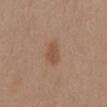notes = no biopsy performed (imaged during a skin exam); lighting = white-light illumination; acquisition = ~15 mm crop, total-body skin-cancer survey; automated lesion analysis = a mean CIELAB color near L≈51 a*≈19 b*≈30, a lesion–skin lightness drop of about 8, and a normalized border contrast of about 6.5; subject = female, approximately 55 years of age; body site = the mid back; lesion size = ≈3 mm.